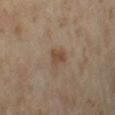  site: left thigh
  patient:
    sex: female
    age_approx: 35
  image:
    source: total-body photography crop
    field_of_view_mm: 15
  lesion_size:
    long_diameter_mm_approx: 2.5
  lighting: cross-polarized
  automated_metrics:
    area_mm2_approx: 4.0
    shape_asymmetry: 0.45
    vs_skin_darker_L: 8.0
    border_irregularity_0_10: 4.0
    nevus_likeness_0_100: 35
    lesion_detection_confidence_0_100: 100